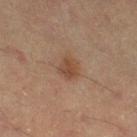* tile lighting · cross-polarized
* acquisition · 15 mm crop, total-body photography
* body site · the leg
* patient · male, roughly 55 years of age